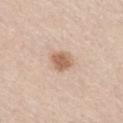The patient is a male aged 58 to 62. A lesion tile, about 15 mm wide, cut from a 3D total-body photograph. Imaged with white-light lighting. The recorded lesion diameter is about 2.5 mm. Located on the right thigh.